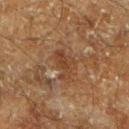Part of a total-body skin-imaging series; this lesion was reviewed on a skin check and was not flagged for biopsy. Captured under cross-polarized illumination. Approximately 4 mm at its widest. The lesion is on the left lower leg. A male subject aged 58–62. Cropped from a total-body skin-imaging series; the visible field is about 15 mm.